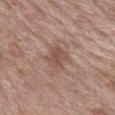Notes:
• location: the right forearm
• diameter: ~3 mm (longest diameter)
• automated lesion analysis: an area of roughly 6 mm², a shape eccentricity near 0.65, and two-axis asymmetry of about 0.2
• patient: male, in their 70s
• image: ~15 mm crop, total-body skin-cancer survey
• illumination: white-light illumination